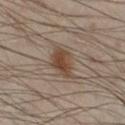biopsy status — total-body-photography surveillance lesion; no biopsy | acquisition — ~15 mm crop, total-body skin-cancer survey | illumination — cross-polarized | subject — male, roughly 50 years of age | lesion diameter — ~3.5 mm (longest diameter) | body site — the leg | automated lesion analysis — a border-irregularity index near 2.5/10, a within-lesion color-variation index near 3/10, and radial color variation of about 1; a nevus-likeness score of about 90/100.